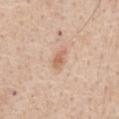| feature | finding |
|---|---|
| notes | imaged on a skin check; not biopsied |